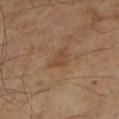Case summary:
- workup: no biopsy performed (imaged during a skin exam)
- image: ~15 mm tile from a whole-body skin photo
- lesion diameter: about 2.5 mm
- patient: male, aged approximately 55
- tile lighting: cross-polarized illumination
- body site: the leg